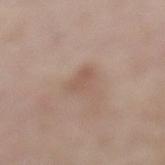Clinical summary:
This is a white-light tile. Automated tile analysis of the lesion measured a border-irregularity index near 3/10, a color-variation rating of about 2/10, and a peripheral color-asymmetry measure near 0.5. It also reported an automated nevus-likeness rating near 0 out of 100 and a detector confidence of about 100 out of 100 that the crop contains a lesion. From the left lower leg. A 15 mm close-up tile from a total-body photography series done for melanoma screening. A female patient, approximately 65 years of age.